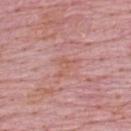A male subject about 65 years old.
This is a white-light tile.
Measured at roughly 3.5 mm in maximum diameter.
The total-body-photography lesion software estimated a lesion area of about 4 mm², a shape eccentricity near 0.75, and two-axis asymmetry of about 0.65. The analysis additionally found a border-irregularity rating of about 7.5/10, a within-lesion color-variation index near 1/10, and peripheral color asymmetry of about 0.5. The analysis additionally found a classifier nevus-likeness of about 0/100.
A close-up tile cropped from a whole-body skin photograph, about 15 mm across.
The lesion is located on the upper back.